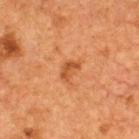• workup · no biopsy performed (imaged during a skin exam)
• patient · male, aged around 50
• tile lighting · cross-polarized illumination
• location · the upper back
• image · 15 mm crop, total-body photography